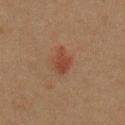image:
  source: total-body photography crop
  field_of_view_mm: 15
automated_metrics:
  color_variation_0_10: 2.5
  peripheral_color_asymmetry: 1.0
site: chest
lighting: cross-polarized
patient:
  sex: male
  age_approx: 40
lesion_size:
  long_diameter_mm_approx: 3.5